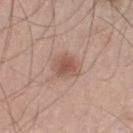This lesion was catalogued during total-body skin photography and was not selected for biopsy. The lesion's longest dimension is about 3.5 mm. A male subject, about 45 years old. A roughly 15 mm field-of-view crop from a total-body skin photograph. Located on the leg.